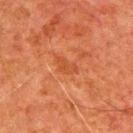Impression: Captured during whole-body skin photography for melanoma surveillance; the lesion was not biopsied. Acquisition and patient details: The lesion is located on the upper back. A close-up tile cropped from a whole-body skin photograph, about 15 mm across. Longest diameter approximately 3 mm. A male subject, in their 80s.